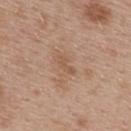Clinical impression:
Part of a total-body skin-imaging series; this lesion was reviewed on a skin check and was not flagged for biopsy.